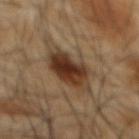imaging modality — ~15 mm crop, total-body skin-cancer survey
location — the mid back
patient — male, aged 63 to 67
automated metrics — an area of roughly 17 mm², an outline eccentricity of about 0.9 (0 = round, 1 = elongated), and a shape-asymmetry score of about 0.2 (0 = symmetric); an automated nevus-likeness rating near 90 out of 100 and a detector confidence of about 100 out of 100 that the crop contains a lesion
diameter — ~8 mm (longest diameter)